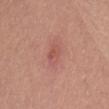Findings:
• follow-up · no biopsy performed (imaged during a skin exam)
• imaging modality · total-body-photography crop, ~15 mm field of view
• size · ~2.5 mm (longest diameter)
• subject · female, in their mid-50s
• location · the leg
• image-analysis metrics · a footprint of about 4 mm², a shape eccentricity near 0.7, and two-axis asymmetry of about 0.25; a mean CIELAB color near L≈54 a*≈26 b*≈27, roughly 7 lightness units darker than nearby skin, and a normalized lesion–skin contrast near 4.5
• illumination · white-light